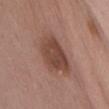  biopsy_status: not biopsied; imaged during a skin examination
  image:
    source: total-body photography crop
    field_of_view_mm: 15
  site: chest
  lighting: white-light
  patient:
    sex: female
    age_approx: 50
  lesion_size:
    long_diameter_mm_approx: 6.5